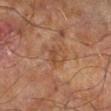Captured during whole-body skin photography for melanoma surveillance; the lesion was not biopsied. A region of skin cropped from a whole-body photographic capture, roughly 15 mm wide. Captured under cross-polarized illumination. A male patient aged 68–72. Longest diameter approximately 3.5 mm. From the right lower leg.